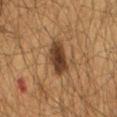| key | value |
|---|---|
| follow-up | catalogued during a skin exam; not biopsied |
| subject | male, roughly 65 years of age |
| image | ~15 mm crop, total-body skin-cancer survey |
| site | the mid back |